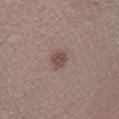biopsy_status: not biopsied; imaged during a skin examination
site: leg
automated_metrics:
  cielab_L: 47
  cielab_a: 18
  cielab_b: 20
  vs_skin_contrast_norm: 7.5
  border_irregularity_0_10: 2.5
  color_variation_0_10: 2.0
  peripheral_color_asymmetry: 0.5
lighting: white-light
patient:
  sex: male
  age_approx: 60
lesion_size:
  long_diameter_mm_approx: 2.5
image:
  source: total-body photography crop
  field_of_view_mm: 15